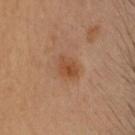Impression:
Imaged during a routine full-body skin examination; the lesion was not biopsied and no histopathology is available.
Clinical summary:
The lesion is located on the head or neck. A female subject, aged approximately 35. This is a cross-polarized tile. Longest diameter approximately 3 mm. A lesion tile, about 15 mm wide, cut from a 3D total-body photograph.